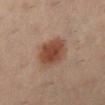<lesion>
  <biopsy_status>not biopsied; imaged during a skin examination</biopsy_status>
  <lighting>cross-polarized</lighting>
  <image>
    <source>total-body photography crop</source>
    <field_of_view_mm>15</field_of_view_mm>
  </image>
  <lesion_size>
    <long_diameter_mm_approx>4.5</long_diameter_mm_approx>
  </lesion_size>
  <patient>
    <sex>female</sex>
    <age_approx>35</age_approx>
  </patient>
  <site>left lower leg</site>
</lesion>